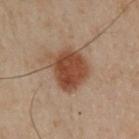Located on the left arm. The patient is a male aged approximately 50. A region of skin cropped from a whole-body photographic capture, roughly 15 mm wide. The total-body-photography lesion software estimated an automated nevus-likeness rating near 100 out of 100 and a detector confidence of about 100 out of 100 that the crop contains a lesion. About 5 mm across. The tile uses cross-polarized illumination.